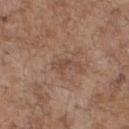This lesion was catalogued during total-body skin photography and was not selected for biopsy. Automated image analysis of the tile measured a lesion area of about 3 mm² and two-axis asymmetry of about 0.45. The analysis additionally found an average lesion color of about L≈47 a*≈18 b*≈27 (CIELAB), roughly 7 lightness units darker than nearby skin, and a normalized lesion–skin contrast near 5.5. The software also gave internal color variation of about 0.5 on a 0–10 scale and radial color variation of about 0. The analysis additionally found lesion-presence confidence of about 100/100. The lesion is located on the chest. The tile uses white-light illumination. The lesion's longest dimension is about 3 mm. The subject is a male in their 70s. Cropped from a whole-body photographic skin survey; the tile spans about 15 mm.